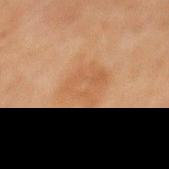Captured during whole-body skin photography for melanoma surveillance; the lesion was not biopsied. A male patient, aged around 50. Approximately 5.5 mm at its widest. The lesion is located on the mid back. This image is a 15 mm lesion crop taken from a total-body photograph.